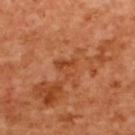  biopsy_status: not biopsied; imaged during a skin examination
  image:
    source: total-body photography crop
    field_of_view_mm: 15
  lighting: cross-polarized
  patient:
    sex: female
    age_approx: 55
  lesion_size:
    long_diameter_mm_approx: 2.5
  site: back